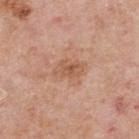The lesion was photographed on a routine skin check and not biopsied; there is no pathology result. The tile uses white-light illumination. A region of skin cropped from a whole-body photographic capture, roughly 15 mm wide. Located on the back. Measured at roughly 3.5 mm in maximum diameter. A male subject, roughly 75 years of age.